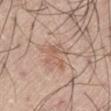Q: Was this lesion biopsied?
A: catalogued during a skin exam; not biopsied
Q: Automated lesion metrics?
A: a lesion color around L≈59 a*≈19 b*≈29 in CIELAB, roughly 7 lightness units darker than nearby skin, and a normalized lesion–skin contrast near 5; a nevus-likeness score of about 30/100 and a detector confidence of about 100 out of 100 that the crop contains a lesion
Q: How was this image acquired?
A: 15 mm crop, total-body photography
Q: Patient demographics?
A: male, about 20 years old
Q: Where on the body is the lesion?
A: the left thigh
Q: Lesion size?
A: ≈3.5 mm